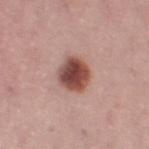Recorded during total-body skin imaging; not selected for excision or biopsy. A region of skin cropped from a whole-body photographic capture, roughly 15 mm wide. About 3.5 mm across. The patient is a female aged approximately 55. The lesion-visualizer software estimated about 17 CIELAB-L* units darker than the surrounding skin and a normalized border contrast of about 12. And it measured border irregularity of about 1.5 on a 0–10 scale. The analysis additionally found a classifier nevus-likeness of about 95/100. The lesion is located on the left thigh. This is a white-light tile.